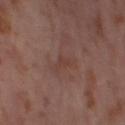• automated metrics: internal color variation of about 0 on a 0–10 scale
• lighting: cross-polarized
• lesion diameter: ≈2.5 mm
• subject: female, in their mid- to late 50s
• body site: the leg
• acquisition: ~15 mm tile from a whole-body skin photo